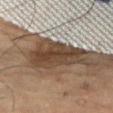follow-up: no biopsy performed (imaged during a skin exam) | subject: male, aged approximately 65 | size: ~7 mm (longest diameter) | image: total-body-photography crop, ~15 mm field of view | body site: the right thigh | image-analysis metrics: a mean CIELAB color near L≈42 a*≈15 b*≈27; a border-irregularity index near 4/10, internal color variation of about 4.5 on a 0–10 scale, and radial color variation of about 2; an automated nevus-likeness rating near 0 out of 100 and a lesion-detection confidence of about 60/100.